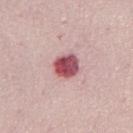Captured during whole-body skin photography for melanoma surveillance; the lesion was not biopsied.
A female subject aged approximately 50.
A roughly 15 mm field-of-view crop from a total-body skin photograph.
The total-body-photography lesion software estimated a footprint of about 7 mm², an eccentricity of roughly 0.35, and a symmetry-axis asymmetry near 0.1. It also reported a lesion color around L≈51 a*≈32 b*≈18 in CIELAB, roughly 20 lightness units darker than nearby skin, and a lesion-to-skin contrast of about 12.5 (normalized; higher = more distinct). And it measured border irregularity of about 1 on a 0–10 scale, a color-variation rating of about 8.5/10, and radial color variation of about 3.
Located on the front of the torso.
Imaged with white-light lighting.